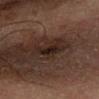A close-up tile cropped from a whole-body skin photograph, about 15 mm across. The tile uses cross-polarized illumination. The total-body-photography lesion software estimated a shape eccentricity near 0.55 and a symmetry-axis asymmetry near 0.45. On the left forearm. A male patient, roughly 65 years of age. Longest diameter approximately 3.5 mm.